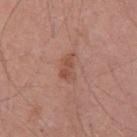notes: catalogued during a skin exam; not biopsied
anatomic site: the chest
acquisition: total-body-photography crop, ~15 mm field of view
TBP lesion metrics: a border-irregularity index near 3.5/10, a within-lesion color-variation index near 2/10, and radial color variation of about 0.5
lighting: white-light illumination
subject: male, aged around 55
diameter: ≈3.5 mm